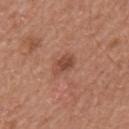{"biopsy_status": "not biopsied; imaged during a skin examination", "lighting": "white-light", "image": {"source": "total-body photography crop", "field_of_view_mm": 15}, "lesion_size": {"long_diameter_mm_approx": 3.0}, "patient": {"sex": "male", "age_approx": 65}, "site": "back", "automated_metrics": {"area_mm2_approx": 4.0, "eccentricity": 0.75, "shape_asymmetry": 0.3, "cielab_L": 47, "cielab_a": 24, "cielab_b": 29, "vs_skin_darker_L": 10.0, "vs_skin_contrast_norm": 7.0}}